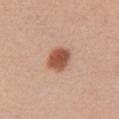{"biopsy_status": "not biopsied; imaged during a skin examination", "patient": {"sex": "female", "age_approx": 40}, "image": {"source": "total-body photography crop", "field_of_view_mm": 15}, "lighting": "white-light", "automated_metrics": {"area_mm2_approx": 7.0, "shape_asymmetry": 0.25, "cielab_L": 53, "cielab_a": 25, "cielab_b": 31, "vs_skin_darker_L": 15.0, "vs_skin_contrast_norm": 10.5, "peripheral_color_asymmetry": 1.0, "nevus_likeness_0_100": 100, "lesion_detection_confidence_0_100": 100}, "site": "chest", "lesion_size": {"long_diameter_mm_approx": 3.0}}